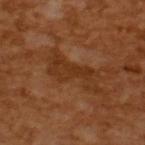Imaged during a routine full-body skin examination; the lesion was not biopsied and no histopathology is available.
Captured under cross-polarized illumination.
About 6.5 mm across.
Cropped from a whole-body photographic skin survey; the tile spans about 15 mm.
The subject is a male aged 63–67.